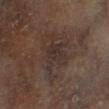{
  "biopsy_status": "not biopsied; imaged during a skin examination",
  "image": {
    "source": "total-body photography crop",
    "field_of_view_mm": 15
  },
  "site": "left lower leg",
  "patient": {
    "sex": "male",
    "age_approx": 65
  },
  "lighting": "cross-polarized",
  "lesion_size": {
    "long_diameter_mm_approx": 6.5
  }
}